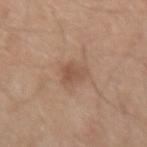Clinical impression:
No biopsy was performed on this lesion — it was imaged during a full skin examination and was not determined to be concerning.
Acquisition and patient details:
The lesion-visualizer software estimated a footprint of about 5 mm² and a shape eccentricity near 0.8. Cropped from a total-body skin-imaging series; the visible field is about 15 mm. Measured at roughly 3 mm in maximum diameter. On the right forearm. Captured under white-light illumination. A male patient, aged around 40.